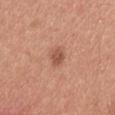Findings:
* notes · no biopsy performed (imaged during a skin exam)
* lesion diameter · ~2.5 mm (longest diameter)
* image · ~15 mm crop, total-body skin-cancer survey
* body site · the head or neck
* lighting · white-light illumination
* patient · male, about 30 years old